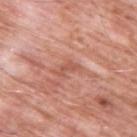workup: total-body-photography surveillance lesion; no biopsy
TBP lesion metrics: an average lesion color of about L≈55 a*≈27 b*≈29 (CIELAB), a lesion–skin lightness drop of about 8, and a lesion-to-skin contrast of about 5.5 (normalized; higher = more distinct); a border-irregularity rating of about 4.5/10, a within-lesion color-variation index near 0/10, and a peripheral color-asymmetry measure near 0; a detector confidence of about 90 out of 100 that the crop contains a lesion
lesion size: ≈3 mm
body site: the upper back
acquisition: 15 mm crop, total-body photography
subject: male, about 60 years old
illumination: white-light